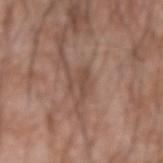Clinical impression: The lesion was tiled from a total-body skin photograph and was not biopsied. Clinical summary: Captured under white-light illumination. About 3 mm across. On the arm. A male patient, approximately 60 years of age. A region of skin cropped from a whole-body photographic capture, roughly 15 mm wide.